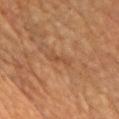notes: no biopsy performed (imaged during a skin exam); TBP lesion metrics: a footprint of about 2.5 mm² and two-axis asymmetry of about 0.45; illumination: cross-polarized illumination; image: ~15 mm tile from a whole-body skin photo; patient: female, in their 70s; size: about 3 mm; anatomic site: the chest.